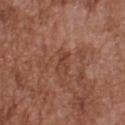Recorded during total-body skin imaging; not selected for excision or biopsy. A male patient, aged 73 to 77. Located on the back. A close-up tile cropped from a whole-body skin photograph, about 15 mm across.